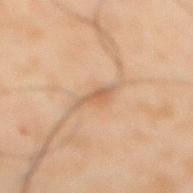{
  "biopsy_status": "not biopsied; imaged during a skin examination",
  "lighting": "cross-polarized",
  "site": "front of the torso",
  "patient": {
    "sex": "male",
    "age_approx": 50
  },
  "image": {
    "source": "total-body photography crop",
    "field_of_view_mm": 15
  }
}